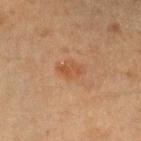Part of a total-body skin-imaging series; this lesion was reviewed on a skin check and was not flagged for biopsy. A 15 mm crop from a total-body photograph taken for skin-cancer surveillance. Located on the left forearm. This is a cross-polarized tile. A female subject, aged 38–42.